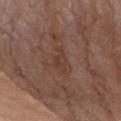Clinical impression: Captured during whole-body skin photography for melanoma surveillance; the lesion was not biopsied. Image and clinical context: The lesion is located on the chest. A 15 mm close-up extracted from a 3D total-body photography capture. A male patient, aged approximately 75.